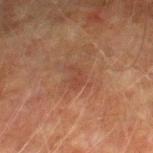The lesion was photographed on a routine skin check and not biopsied; there is no pathology result.